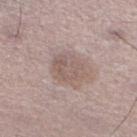The lesion was tiled from a total-body skin photograph and was not biopsied. The recorded lesion diameter is about 4 mm. A male subject approximately 45 years of age. A 15 mm close-up extracted from a 3D total-body photography capture. Located on the right lower leg.